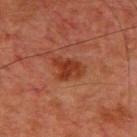follow-up = imaged on a skin check; not biopsied | image source = total-body-photography crop, ~15 mm field of view | body site = the chest | patient = male, aged 58–62.